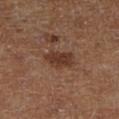The lesion was photographed on a routine skin check and not biopsied; there is no pathology result. Measured at roughly 3.5 mm in maximum diameter. A female subject. Located on the right lower leg. A region of skin cropped from a whole-body photographic capture, roughly 15 mm wide. Imaged with cross-polarized lighting.